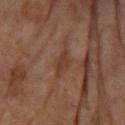| feature | finding |
|---|---|
| image | 15 mm crop, total-body photography |
| illumination | cross-polarized |
| automated metrics | roughly 6 lightness units darker than nearby skin and a normalized border contrast of about 6.5; a border-irregularity rating of about 3/10, a within-lesion color-variation index near 0.5/10, and radial color variation of about 0; a nevus-likeness score of about 0/100 |
| subject | female, aged around 80 |
| location | the arm |